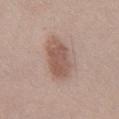  lighting: white-light
  patient:
    sex: male
    age_approx: 40
  site: abdomen
  automated_metrics:
    cielab_L: 55
    cielab_a: 19
    cielab_b: 25
    vs_skin_darker_L: 11.0
    vs_skin_contrast_norm: 7.5
  image:
    source: total-body photography crop
    field_of_view_mm: 15